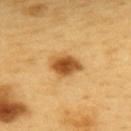Assessment: Recorded during total-body skin imaging; not selected for excision or biopsy. Image and clinical context: A region of skin cropped from a whole-body photographic capture, roughly 15 mm wide. The lesion is located on the back. The subject is a male aged 58 to 62.